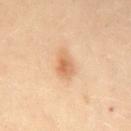| key | value |
|---|---|
| notes | total-body-photography surveillance lesion; no biopsy |
| site | the mid back |
| image | ~15 mm crop, total-body skin-cancer survey |
| patient | female, approximately 30 years of age |
| lesion diameter | about 3 mm |